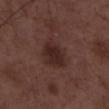Clinical impression:
Part of a total-body skin-imaging series; this lesion was reviewed on a skin check and was not flagged for biopsy.
Context:
A male patient, aged 48–52. A roughly 15 mm field-of-view crop from a total-body skin photograph.